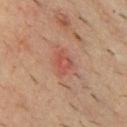Captured during whole-body skin photography for melanoma surveillance; the lesion was not biopsied.